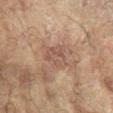• follow-up · imaged on a skin check; not biopsied
• subject · female, aged 78–82
• diameter · ~3.5 mm (longest diameter)
• image-analysis metrics · a lesion area of about 7.5 mm² and a shape eccentricity near 0.55; a lesion color around L≈48 a*≈17 b*≈25 in CIELAB, roughly 6 lightness units darker than nearby skin, and a normalized border contrast of about 5; an automated nevus-likeness rating near 0 out of 100 and a lesion-detection confidence of about 100/100
• location · the right arm
• imaging modality · ~15 mm crop, total-body skin-cancer survey
• illumination · cross-polarized illumination